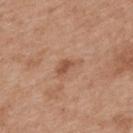Recorded during total-body skin imaging; not selected for excision or biopsy.